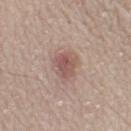No biopsy was performed on this lesion — it was imaged during a full skin examination and was not determined to be concerning.
The subject is a female in their 60s.
From the right lower leg.
A roughly 15 mm field-of-view crop from a total-body skin photograph.